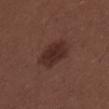follow-up = catalogued during a skin exam; not biopsied | acquisition = total-body-photography crop, ~15 mm field of view | lighting = white-light illumination | subject = male, aged approximately 30 | location = the upper back | diameter = ~5 mm (longest diameter).